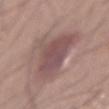workup = total-body-photography surveillance lesion; no biopsy | image source = ~15 mm crop, total-body skin-cancer survey | anatomic site = the abdomen | illumination = white-light | patient = male, aged 53–57.